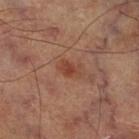Findings:
* anatomic site · the right thigh
* illumination · cross-polarized illumination
* image source · ~15 mm tile from a whole-body skin photo